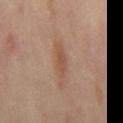The lesion was photographed on a routine skin check and not biopsied; there is no pathology result. Located on the chest. A region of skin cropped from a whole-body photographic capture, roughly 15 mm wide. This is a cross-polarized tile. A female subject roughly 50 years of age.